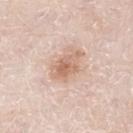- illumination: white-light illumination
- size: ≈4.5 mm
- acquisition: 15 mm crop, total-body photography
- body site: the left lower leg
- patient: male, aged approximately 80